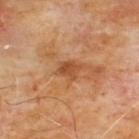Clinical impression:
Recorded during total-body skin imaging; not selected for excision or biopsy.
Image and clinical context:
Longest diameter approximately 2.5 mm. The lesion is on the chest. Captured under cross-polarized illumination. A male patient aged around 60. A 15 mm close-up tile from a total-body photography series done for melanoma screening.